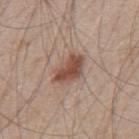– follow-up — total-body-photography surveillance lesion; no biopsy
– TBP lesion metrics — a footprint of about 9 mm² and an outline eccentricity of about 0.8 (0 = round, 1 = elongated)
– illumination — white-light
– size — ~4.5 mm (longest diameter)
– site — the mid back
– image source — ~15 mm crop, total-body skin-cancer survey
– patient — male, in their 50s